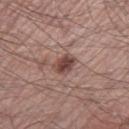Recorded during total-body skin imaging; not selected for excision or biopsy.
The lesion is on the right thigh.
About 2.5 mm across.
A roughly 15 mm field-of-view crop from a total-body skin photograph.
The patient is a male aged 53–57.